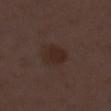Impression:
The lesion was photographed on a routine skin check and not biopsied; there is no pathology result.
Image and clinical context:
A region of skin cropped from a whole-body photographic capture, roughly 15 mm wide. Automated tile analysis of the lesion measured a lesion area of about 7 mm². The lesion is on the right lower leg. The patient is a female aged 48 to 52.